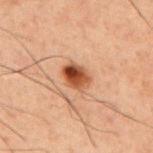Case summary:
- follow-up — no biopsy performed (imaged during a skin exam)
- anatomic site — the upper back
- lesion diameter — about 3 mm
- lighting — cross-polarized illumination
- automated metrics — a footprint of about 6.5 mm², an eccentricity of roughly 0.7, and two-axis asymmetry of about 0.2; an average lesion color of about L≈39 a*≈21 b*≈30 (CIELAB) and roughly 13 lightness units darker than nearby skin; border irregularity of about 2 on a 0–10 scale, a color-variation rating of about 9/10, and peripheral color asymmetry of about 4; a classifier nevus-likeness of about 100/100 and a detector confidence of about 100 out of 100 that the crop contains a lesion
- patient — male, aged 58 to 62
- imaging modality — 15 mm crop, total-body photography